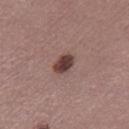The lesion was photographed on a routine skin check and not biopsied; there is no pathology result. Imaged with white-light lighting. A lesion tile, about 15 mm wide, cut from a 3D total-body photograph. Approximately 2.5 mm at its widest. The total-body-photography lesion software estimated a lesion area of about 4.5 mm², an eccentricity of roughly 0.65, and two-axis asymmetry of about 0.25. The software also gave a lesion color around L≈39 a*≈20 b*≈20 in CIELAB and roughly 15 lightness units darker than nearby skin. The software also gave border irregularity of about 2 on a 0–10 scale and a color-variation rating of about 4.5/10. The software also gave a nevus-likeness score of about 90/100 and lesion-presence confidence of about 100/100. The lesion is on the right thigh. The subject is a female aged 48 to 52.